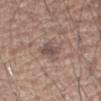Captured during whole-body skin photography for melanoma surveillance; the lesion was not biopsied. The total-body-photography lesion software estimated a lesion area of about 6 mm² and a symmetry-axis asymmetry near 0.3. The analysis additionally found a normalized lesion–skin contrast near 7. The analysis additionally found a border-irregularity index near 3/10, a within-lesion color-variation index near 2/10, and a peripheral color-asymmetry measure near 0.5. It also reported a classifier nevus-likeness of about 0/100 and lesion-presence confidence of about 95/100. Captured under white-light illumination. Located on the right forearm. This image is a 15 mm lesion crop taken from a total-body photograph. A male patient, approximately 65 years of age.